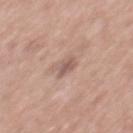Q: Was a biopsy performed?
A: total-body-photography surveillance lesion; no biopsy
Q: Lesion size?
A: about 2.5 mm
Q: What kind of image is this?
A: ~15 mm tile from a whole-body skin photo
Q: How was the tile lit?
A: white-light
Q: Automated lesion metrics?
A: a normalized border contrast of about 7; internal color variation of about 2 on a 0–10 scale and peripheral color asymmetry of about 0.5; an automated nevus-likeness rating near 0 out of 100 and lesion-presence confidence of about 100/100
Q: Who is the patient?
A: male, roughly 70 years of age
Q: What is the anatomic site?
A: the mid back